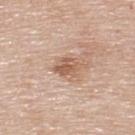Impression: This lesion was catalogued during total-body skin photography and was not selected for biopsy. Clinical summary: A male subject, aged 78–82. Captured under white-light illumination. A 15 mm crop from a total-body photograph taken for skin-cancer surveillance. On the back. Measured at roughly 3 mm in maximum diameter.